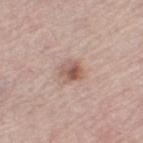<case>
  <biopsy_status>not biopsied; imaged during a skin examination</biopsy_status>
  <lighting>white-light</lighting>
  <site>right thigh</site>
  <patient>
    <sex>female</sex>
    <age_approx>65</age_approx>
  </patient>
  <image>
    <source>total-body photography crop</source>
    <field_of_view_mm>15</field_of_view_mm>
  </image>
</case>